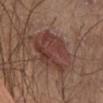The lesion was photographed on a routine skin check and not biopsied; there is no pathology result. The lesion is on the chest. A male patient approximately 65 years of age. Automated image analysis of the tile measured a footprint of about 23 mm², an outline eccentricity of about 0.7 (0 = round, 1 = elongated), and two-axis asymmetry of about 0.2. The software also gave an average lesion color of about L≈39 a*≈21 b*≈23 (CIELAB), about 8 CIELAB-L* units darker than the surrounding skin, and a lesion-to-skin contrast of about 7.5 (normalized; higher = more distinct). It also reported a classifier nevus-likeness of about 35/100 and lesion-presence confidence of about 100/100. Captured under white-light illumination. Approximately 7 mm at its widest. A close-up tile cropped from a whole-body skin photograph, about 15 mm across.